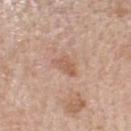This lesion was catalogued during total-body skin photography and was not selected for biopsy.
A female subject, about 60 years old.
A 15 mm crop from a total-body photograph taken for skin-cancer surveillance.
Located on the head or neck.
An algorithmic analysis of the crop reported an eccentricity of roughly 0.85. The software also gave a border-irregularity index near 4/10.
Captured under white-light illumination.